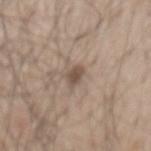Part of a total-body skin-imaging series; this lesion was reviewed on a skin check and was not flagged for biopsy. The lesion is located on the mid back. A roughly 15 mm field-of-view crop from a total-body skin photograph. A male subject, aged around 50.